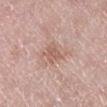<record>
<image>
  <source>total-body photography crop</source>
  <field_of_view_mm>15</field_of_view_mm>
</image>
<lighting>white-light</lighting>
<site>left lower leg</site>
<automated_metrics>
  <area_mm2_approx>4.5</area_mm2_approx>
  <eccentricity>0.75</eccentricity>
  <shape_asymmetry>0.35</shape_asymmetry>
  <nevus_likeness_0_100>0</nevus_likeness_0_100>
  <lesion_detection_confidence_0_100>100</lesion_detection_confidence_0_100>
</automated_metrics>
<patient>
  <sex>female</sex>
  <age_approx>65</age_approx>
</patient>
</record>